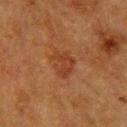This lesion was catalogued during total-body skin photography and was not selected for biopsy.